A male patient about 45 years old; approximately 4.5 mm at its widest; located on the abdomen; Automated image analysis of the tile measured a border-irregularity index near 5/10 and a peripheral color-asymmetry measure near 2. The analysis additionally found a nevus-likeness score of about 0/100 and a detector confidence of about 0 out of 100 that the crop contains a lesion; a roughly 15 mm field-of-view crop from a total-body skin photograph; imaged with cross-polarized lighting — 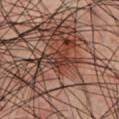On biopsy, histopathology showed a dysplastic (Clark) nevus.